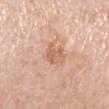<lesion>
  <biopsy_status>not biopsied; imaged during a skin examination</biopsy_status>
  <image>
    <source>total-body photography crop</source>
    <field_of_view_mm>15</field_of_view_mm>
  </image>
  <automated_metrics>
    <cielab_L>63</cielab_L>
    <cielab_a>22</cielab_a>
    <cielab_b>32</cielab_b>
    <vs_skin_contrast_norm>6.0</vs_skin_contrast_norm>
    <nevus_likeness_0_100>0</nevus_likeness_0_100>
    <lesion_detection_confidence_0_100>100</lesion_detection_confidence_0_100>
  </automated_metrics>
  <lesion_size>
    <long_diameter_mm_approx>3.0</long_diameter_mm_approx>
  </lesion_size>
  <lighting>white-light</lighting>
  <site>left lower leg</site>
  <patient>
    <sex>female</sex>
    <age_approx>70</age_approx>
  </patient>
</lesion>